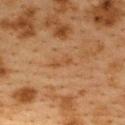biopsy status = catalogued during a skin exam; not biopsied | image = 15 mm crop, total-body photography | subject = female, aged approximately 40 | illumination = cross-polarized | diameter = ≈2.5 mm | anatomic site = the upper back.